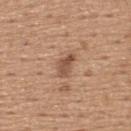This lesion was catalogued during total-body skin photography and was not selected for biopsy. This is a white-light tile. A region of skin cropped from a whole-body photographic capture, roughly 15 mm wide. The lesion's longest dimension is about 3 mm. From the upper back. A male subject approximately 65 years of age. Automated image analysis of the tile measured a lesion area of about 4.5 mm² and a symmetry-axis asymmetry near 0.35. It also reported a mean CIELAB color near L≈52 a*≈20 b*≈30, roughly 11 lightness units darker than nearby skin, and a lesion-to-skin contrast of about 7.5 (normalized; higher = more distinct). It also reported border irregularity of about 3 on a 0–10 scale and a peripheral color-asymmetry measure near 1.5.